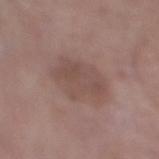No biopsy was performed on this lesion — it was imaged during a full skin examination and was not determined to be concerning. A roughly 15 mm field-of-view crop from a total-body skin photograph. This is a white-light tile. The total-body-photography lesion software estimated a mean CIELAB color near L≈48 a*≈18 b*≈22, a lesion–skin lightness drop of about 7, and a lesion-to-skin contrast of about 5.5 (normalized; higher = more distinct). And it measured a color-variation rating of about 2.5/10 and a peripheral color-asymmetry measure near 1. The analysis additionally found an automated nevus-likeness rating near 0 out of 100 and lesion-presence confidence of about 100/100. The lesion is located on the right lower leg. A female subject, aged 83 or older. The lesion's longest dimension is about 5.5 mm.